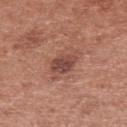Part of a total-body skin-imaging series; this lesion was reviewed on a skin check and was not flagged for biopsy. A 15 mm close-up tile from a total-body photography series done for melanoma screening. The lesion-visualizer software estimated an outline eccentricity of about 0.5 (0 = round, 1 = elongated) and a shape-asymmetry score of about 0.25 (0 = symmetric). The software also gave a border-irregularity rating of about 2/10, a within-lesion color-variation index near 3/10, and a peripheral color-asymmetry measure near 1. The software also gave an automated nevus-likeness rating near 45 out of 100 and a lesion-detection confidence of about 100/100. Approximately 3 mm at its widest. On the upper back. A male patient, aged 53 to 57. Captured under white-light illumination.